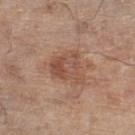The subject is a male aged 78 to 82. Located on the left lower leg. A roughly 15 mm field-of-view crop from a total-body skin photograph. This is a white-light tile. Longest diameter approximately 4.5 mm. The lesion-visualizer software estimated a footprint of about 10 mm² and a symmetry-axis asymmetry near 0.35. The analysis additionally found a border-irregularity index near 4/10 and a within-lesion color-variation index near 6/10. The analysis additionally found a nevus-likeness score of about 25/100 and a lesion-detection confidence of about 100/100.